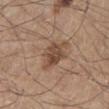Case summary:
• biopsy status — imaged on a skin check; not biopsied
• subject — male, in their mid-40s
• imaging modality — ~15 mm crop, total-body skin-cancer survey
• illumination — white-light
• diameter — ~4 mm (longest diameter)
• automated lesion analysis — an area of roughly 9 mm² and a shape-asymmetry score of about 0.3 (0 = symmetric); border irregularity of about 3.5 on a 0–10 scale, a color-variation rating of about 4/10, and radial color variation of about 1.5
• site — the left lower leg